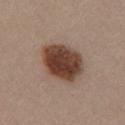  automated_metrics:
    area_mm2_approx: 19.0
    eccentricity: 0.65
    vs_skin_darker_L: 17.0
    vs_skin_contrast_norm: 12.5
    nevus_likeness_0_100: 100
    lesion_detection_confidence_0_100: 100
  lesion_size:
    long_diameter_mm_approx: 5.5
  site: chest
  patient:
    sex: female
    age_approx: 45
  image:
    source: total-body photography crop
    field_of_view_mm: 15
  lighting: white-light